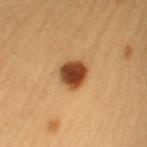follow-up — imaged on a skin check; not biopsied
size — about 3 mm
image — 15 mm crop, total-body photography
site — the left upper arm
patient — female, aged 43–47
tile lighting — cross-polarized illumination